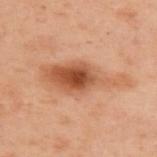workup = imaged on a skin check; not biopsied | body site = the upper back | imaging modality = ~15 mm tile from a whole-body skin photo | patient = female, aged approximately 55.